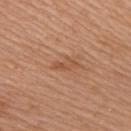Q: Is there a histopathology result?
A: no biopsy performed (imaged during a skin exam)
Q: What is the imaging modality?
A: ~15 mm crop, total-body skin-cancer survey
Q: How large is the lesion?
A: ~2.5 mm (longest diameter)
Q: Automated lesion metrics?
A: a footprint of about 3 mm², an outline eccentricity of about 0.9 (0 = round, 1 = elongated), and a symmetry-axis asymmetry near 0.4
Q: What is the anatomic site?
A: the right upper arm
Q: Who is the patient?
A: female, aged approximately 60